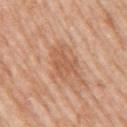Clinical impression: Part of a total-body skin-imaging series; this lesion was reviewed on a skin check and was not flagged for biopsy. Acquisition and patient details: This is a white-light tile. A female subject aged 68 to 72. On the right upper arm. A 15 mm close-up extracted from a 3D total-body photography capture.